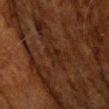Q: Was a biopsy performed?
A: no biopsy performed (imaged during a skin exam)
Q: What kind of image is this?
A: 15 mm crop, total-body photography
Q: What did automated image analysis measure?
A: a lesion area of about 4 mm², an eccentricity of roughly 0.75, and a shape-asymmetry score of about 0.3 (0 = symmetric)
Q: What lighting was used for the tile?
A: cross-polarized
Q: What is the lesion's diameter?
A: about 2.5 mm
Q: What is the anatomic site?
A: the head or neck
Q: Who is the patient?
A: male, aged around 80